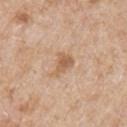notes=imaged on a skin check; not biopsied | illumination=white-light | size=≈3 mm | patient=male, aged approximately 65 | location=the right upper arm | image source=~15 mm tile from a whole-body skin photo.